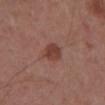Case summary:
* biopsy status: catalogued during a skin exam; not biopsied
* location: the abdomen
* automated lesion analysis: a lesion color around L≈40 a*≈23 b*≈26 in CIELAB, roughly 9 lightness units darker than nearby skin, and a lesion-to-skin contrast of about 8 (normalized; higher = more distinct); a border-irregularity rating of about 1.5/10 and internal color variation of about 2.5 on a 0–10 scale; a nevus-likeness score of about 85/100 and a lesion-detection confidence of about 100/100
* patient: male, in their mid- to late 50s
* acquisition: ~15 mm tile from a whole-body skin photo
* lesion size: ≈2.5 mm
* tile lighting: white-light illumination